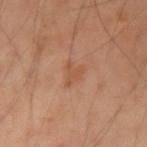Findings:
* biopsy status — no biopsy performed (imaged during a skin exam)
* anatomic site — the arm
* lesion size — ~2.5 mm (longest diameter)
* imaging modality — total-body-photography crop, ~15 mm field of view
* tile lighting — cross-polarized illumination
* subject — male, about 45 years old